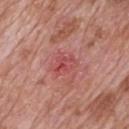Assessment:
The lesion was photographed on a routine skin check and not biopsied; there is no pathology result.